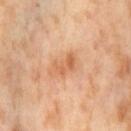- biopsy status: total-body-photography surveillance lesion; no biopsy
- image: total-body-photography crop, ~15 mm field of view
- automated lesion analysis: an eccentricity of roughly 0.85 and a shape-asymmetry score of about 0.4 (0 = symmetric); a lesion color around L≈64 a*≈25 b*≈39 in CIELAB and a normalized border contrast of about 6; a border-irregularity index near 4.5/10 and radial color variation of about 1.5; a lesion-detection confidence of about 100/100
- tile lighting: cross-polarized
- body site: the left thigh
- subject: female, aged 53 to 57